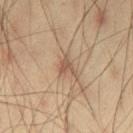Captured during whole-body skin photography for melanoma surveillance; the lesion was not biopsied.
A roughly 15 mm field-of-view crop from a total-body skin photograph.
The subject is a male aged around 40.
Located on the left thigh.